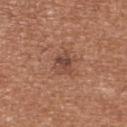{
  "biopsy_status": "not biopsied; imaged during a skin examination",
  "patient": {
    "sex": "female",
    "age_approx": 40
  },
  "image": {
    "source": "total-body photography crop",
    "field_of_view_mm": 15
  },
  "lesion_size": {
    "long_diameter_mm_approx": 3.0
  },
  "lighting": "white-light",
  "site": "upper back",
  "automated_metrics": {
    "eccentricity": 0.65,
    "shape_asymmetry": 0.25,
    "nevus_likeness_0_100": 25,
    "lesion_detection_confidence_0_100": 100
  }
}